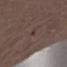The lesion was tiled from a total-body skin photograph and was not biopsied. A 15 mm close-up extracted from a 3D total-body photography capture. From the right upper arm. A male patient aged approximately 70.